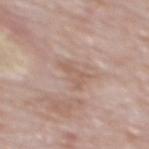The lesion was tiled from a total-body skin photograph and was not biopsied.
The lesion is on the mid back.
The tile uses white-light illumination.
Longest diameter approximately 3 mm.
The subject is a male approximately 75 years of age.
A lesion tile, about 15 mm wide, cut from a 3D total-body photograph.